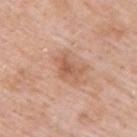- follow-up · total-body-photography surveillance lesion; no biopsy
- body site · the upper back
- patient · male, about 55 years old
- acquisition · ~15 mm crop, total-body skin-cancer survey
- diameter · ~3.5 mm (longest diameter)
- TBP lesion metrics · a lesion color around L≈58 a*≈23 b*≈32 in CIELAB and a lesion–skin lightness drop of about 9; a border-irregularity index near 5.5/10 and a peripheral color-asymmetry measure near 1